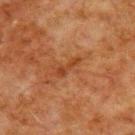biopsy status = catalogued during a skin exam; not biopsied
illumination = cross-polarized illumination
location = the upper back
size = ≈3.5 mm
subject = male, approximately 80 years of age
TBP lesion metrics = lesion-presence confidence of about 100/100
image source = ~15 mm crop, total-body skin-cancer survey